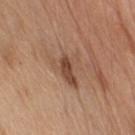Context: This image is a 15 mm lesion crop taken from a total-body photograph. Automated tile analysis of the lesion measured an eccentricity of roughly 0.6 and two-axis asymmetry of about 0.5. And it measured a border-irregularity rating of about 6/10, a within-lesion color-variation index near 6/10, and peripheral color asymmetry of about 2. The lesion is on the left upper arm. A male subject aged 68–72. Imaged with white-light lighting. The recorded lesion diameter is about 5 mm.